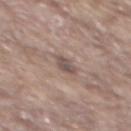Impression:
Part of a total-body skin-imaging series; this lesion was reviewed on a skin check and was not flagged for biopsy.
Clinical summary:
A male patient, roughly 65 years of age. A 15 mm close-up extracted from a 3D total-body photography capture. On the mid back.